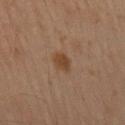{"biopsy_status": "not biopsied; imaged during a skin examination", "lighting": "cross-polarized", "image": {"source": "total-body photography crop", "field_of_view_mm": 15}, "patient": {"sex": "male", "age_approx": 60}, "site": "right thigh", "automated_metrics": {"vs_skin_darker_L": 7.0, "vs_skin_contrast_norm": 7.0, "color_variation_0_10": 1.5, "peripheral_color_asymmetry": 0.5}}